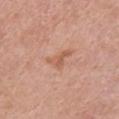biopsy_status: not biopsied; imaged during a skin examination
patient:
  sex: male
  age_approx: 60
site: front of the torso
image:
  source: total-body photography crop
  field_of_view_mm: 15